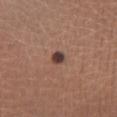Assessment:
Part of a total-body skin-imaging series; this lesion was reviewed on a skin check and was not flagged for biopsy.
Context:
The tile uses white-light illumination. Automated image analysis of the tile measured an area of roughly 3 mm², an outline eccentricity of about 0.5 (0 = round, 1 = elongated), and a shape-asymmetry score of about 0.3 (0 = symmetric). And it measured a lesion color around L≈39 a*≈19 b*≈21 in CIELAB. It also reported a border-irregularity index near 2.5/10, a color-variation rating of about 3.5/10, and radial color variation of about 1. And it measured a nevus-likeness score of about 85/100 and lesion-presence confidence of about 100/100. A female patient aged 33 to 37. The lesion is located on the right lower leg. A 15 mm close-up extracted from a 3D total-body photography capture. Approximately 2 mm at its widest.